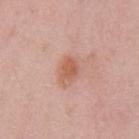{
  "biopsy_status": "not biopsied; imaged during a skin examination",
  "site": "arm",
  "automated_metrics": {
    "area_mm2_approx": 4.0,
    "eccentricity": 0.65,
    "shape_asymmetry": 0.25,
    "border_irregularity_0_10": 2.5,
    "color_variation_0_10": 1.5,
    "peripheral_color_asymmetry": 0.5,
    "nevus_likeness_0_100": 85,
    "lesion_detection_confidence_0_100": 100
  },
  "patient": {
    "sex": "female",
    "age_approx": 25
  },
  "lighting": "white-light",
  "image": {
    "source": "total-body photography crop",
    "field_of_view_mm": 15
  },
  "lesion_size": {
    "long_diameter_mm_approx": 2.5
  }
}